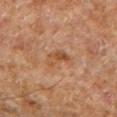workup = total-body-photography surveillance lesion; no biopsy
image source = ~15 mm crop, total-body skin-cancer survey
location = the left forearm
automated metrics = an average lesion color of about L≈45 a*≈20 b*≈32 (CIELAB), about 7 CIELAB-L* units darker than the surrounding skin, and a normalized border contrast of about 6.5; a border-irregularity rating of about 3.5/10, internal color variation of about 5.5 on a 0–10 scale, and radial color variation of about 2.5
lighting = cross-polarized
subject = male, aged 63 to 67